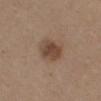workup=no biopsy performed (imaged during a skin exam) | image source=15 mm crop, total-body photography | location=the chest | patient=female, aged around 40.